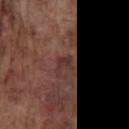  biopsy_status: not biopsied; imaged during a skin examination
  image:
    source: total-body photography crop
    field_of_view_mm: 15
  lighting: white-light
  lesion_size:
    long_diameter_mm_approx: 2.5
  site: chest
  patient:
    sex: male
    age_approx: 75